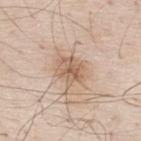<tbp_lesion>
  <biopsy_status>not biopsied; imaged during a skin examination</biopsy_status>
  <lighting>white-light</lighting>
  <site>upper back</site>
  <automated_metrics>
    <area_mm2_approx>7.5</area_mm2_approx>
    <eccentricity>0.55</eccentricity>
    <cielab_L>61</cielab_L>
    <cielab_a>17</cielab_a>
    <cielab_b>30</cielab_b>
    <vs_skin_darker_L>10.0</vs_skin_darker_L>
    <vs_skin_contrast_norm>7.0</vs_skin_contrast_norm>
    <nevus_likeness_0_100>25</nevus_likeness_0_100>
  </automated_metrics>
  <patient>
    <sex>male</sex>
    <age_approx>80</age_approx>
  </patient>
  <lesion_size>
    <long_diameter_mm_approx>3.5</long_diameter_mm_approx>
  </lesion_size>
  <image>
    <source>total-body photography crop</source>
    <field_of_view_mm>15</field_of_view_mm>
  </image>
</tbp_lesion>